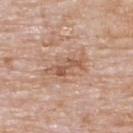The lesion was tiled from a total-body skin photograph and was not biopsied. Cropped from a whole-body photographic skin survey; the tile spans about 15 mm. A male patient, aged approximately 75. Located on the upper back.